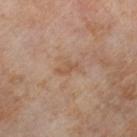No biopsy was performed on this lesion — it was imaged during a full skin examination and was not determined to be concerning. The total-body-photography lesion software estimated a shape eccentricity near 0.9 and a symmetry-axis asymmetry near 0.55. The software also gave a border-irregularity index near 6.5/10, a color-variation rating of about 0/10, and a peripheral color-asymmetry measure near 0. And it measured an automated nevus-likeness rating near 0 out of 100. Located on the right lower leg. A female patient aged 53 to 57. Measured at roughly 2.5 mm in maximum diameter. Imaged with cross-polarized lighting. This image is a 15 mm lesion crop taken from a total-body photograph.